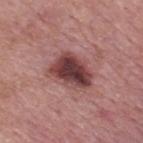A 15 mm close-up extracted from a 3D total-body photography capture. Located on the upper back. Captured under white-light illumination. The lesion-visualizer software estimated a lesion area of about 14 mm² and an eccentricity of roughly 0.75. The analysis additionally found an average lesion color of about L≈41 a*≈25 b*≈20 (CIELAB), a lesion–skin lightness drop of about 16, and a lesion-to-skin contrast of about 12 (normalized; higher = more distinct). Measured at roughly 5 mm in maximum diameter. The patient is a male aged 53 to 57. The biopsy diagnosis was a dysplastic (Clark) nevus — a benign skin lesion.